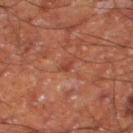Q: Was a biopsy performed?
A: imaged on a skin check; not biopsied
Q: What are the patient's age and sex?
A: male, in their 60s
Q: Illumination type?
A: cross-polarized
Q: Automated lesion metrics?
A: a lesion area of about 1 mm², an outline eccentricity of about 0.65 (0 = round, 1 = elongated), and a symmetry-axis asymmetry near 0.5; a mean CIELAB color near L≈38 a*≈28 b*≈30 and a lesion-to-skin contrast of about 6.5 (normalized; higher = more distinct)
Q: Lesion location?
A: the right thigh
Q: How was this image acquired?
A: ~15 mm crop, total-body skin-cancer survey
Q: Lesion size?
A: about 1.5 mm